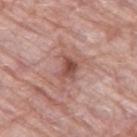Assessment: The lesion was tiled from a total-body skin photograph and was not biopsied. Image and clinical context: The tile uses white-light illumination. The lesion is located on the leg. An algorithmic analysis of the crop reported an eccentricity of roughly 0.7 and a shape-asymmetry score of about 0.35 (0 = symmetric). And it measured roughly 11 lightness units darker than nearby skin and a normalized lesion–skin contrast near 7.5. A close-up tile cropped from a whole-body skin photograph, about 15 mm across. A female subject, aged 68 to 72.